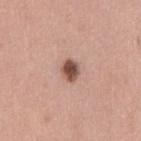Captured during whole-body skin photography for melanoma surveillance; the lesion was not biopsied. The tile uses white-light illumination. A female patient, in their 50s. The lesion-visualizer software estimated a lesion area of about 4 mm², an eccentricity of roughly 0.7, and a symmetry-axis asymmetry near 0.15. It also reported about 17 CIELAB-L* units darker than the surrounding skin and a normalized border contrast of about 11.5. The analysis additionally found internal color variation of about 4 on a 0–10 scale and peripheral color asymmetry of about 1. It also reported an automated nevus-likeness rating near 100 out of 100 and lesion-presence confidence of about 100/100. From the left thigh. The recorded lesion diameter is about 2.5 mm. A region of skin cropped from a whole-body photographic capture, roughly 15 mm wide.